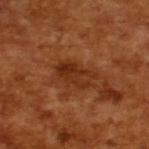* notes: no biopsy performed (imaged during a skin exam)
* subject: male, approximately 65 years of age
* imaging modality: total-body-photography crop, ~15 mm field of view